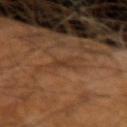Clinical impression:
The lesion was tiled from a total-body skin photograph and was not biopsied.
Background:
The patient is a male about 65 years old. A lesion tile, about 15 mm wide, cut from a 3D total-body photograph. Automated tile analysis of the lesion measured an average lesion color of about L≈35 a*≈19 b*≈30 (CIELAB), a lesion–skin lightness drop of about 6, and a normalized border contrast of about 5.5. The software also gave a classifier nevus-likeness of about 0/100 and a detector confidence of about 80 out of 100 that the crop contains a lesion. Captured under cross-polarized illumination. Measured at roughly 2.5 mm in maximum diameter.